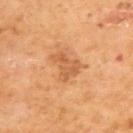Image and clinical context: Cropped from a whole-body photographic skin survey; the tile spans about 15 mm. A male subject, approximately 65 years of age. Captured under cross-polarized illumination. Located on the back. Longest diameter approximately 3.5 mm.